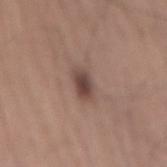Part of a total-body skin-imaging series; this lesion was reviewed on a skin check and was not flagged for biopsy.
A female subject, roughly 65 years of age.
Cropped from a total-body skin-imaging series; the visible field is about 15 mm.
The lesion is located on the mid back.